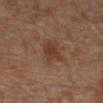The lesion was photographed on a routine skin check and not biopsied; there is no pathology result.
A roughly 15 mm field-of-view crop from a total-body skin photograph.
The subject is a male in their 30s.
The lesion is located on the left leg.